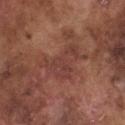Impression: Recorded during total-body skin imaging; not selected for excision or biopsy. Clinical summary: A lesion tile, about 15 mm wide, cut from a 3D total-body photograph. The patient is a male approximately 75 years of age. The lesion is on the front of the torso.